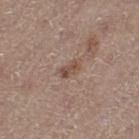<case>
<biopsy_status>not biopsied; imaged during a skin examination</biopsy_status>
<image>
  <source>total-body photography crop</source>
  <field_of_view_mm>15</field_of_view_mm>
</image>
<site>left thigh</site>
<patient>
  <sex>female</sex>
  <age_approx>50</age_approx>
</patient>
<lighting>white-light</lighting>
</case>